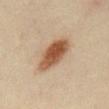This lesion was catalogued during total-body skin photography and was not selected for biopsy. Imaged with cross-polarized lighting. A roughly 15 mm field-of-view crop from a total-body skin photograph. The patient is a male in their 50s. Approximately 5.5 mm at its widest. From the chest.